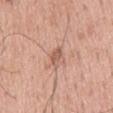The lesion was tiled from a total-body skin photograph and was not biopsied. Imaged with white-light lighting. Automated image analysis of the tile measured an area of roughly 3 mm², a shape eccentricity near 0.9, and a shape-asymmetry score of about 0.35 (0 = symmetric). The analysis additionally found a normalized lesion–skin contrast near 6.5. The analysis additionally found a nevus-likeness score of about 0/100 and lesion-presence confidence of about 100/100. A male subject aged approximately 60. On the mid back. This image is a 15 mm lesion crop taken from a total-body photograph. About 3 mm across.